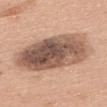image-analysis metrics: an area of roughly 42 mm² and an eccentricity of roughly 0.85; a lesion color around L≈55 a*≈18 b*≈27 in CIELAB, about 17 CIELAB-L* units darker than the surrounding skin, and a normalized lesion–skin contrast near 11; a classifier nevus-likeness of about 10/100 and lesion-presence confidence of about 100/100
imaging modality: total-body-photography crop, ~15 mm field of view
patient: female, about 60 years old
illumination: white-light illumination
anatomic site: the upper back